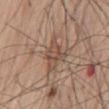Q: Was this lesion biopsied?
A: total-body-photography surveillance lesion; no biopsy
Q: How was the tile lit?
A: white-light illumination
Q: Patient demographics?
A: male, aged 68 to 72
Q: What is the imaging modality?
A: total-body-photography crop, ~15 mm field of view
Q: What is the lesion's diameter?
A: about 4 mm
Q: What is the anatomic site?
A: the mid back